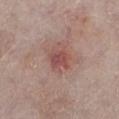Clinical impression: The lesion was photographed on a routine skin check and not biopsied; there is no pathology result. Context: The lesion is located on the left lower leg. The tile uses white-light illumination. A 15 mm close-up extracted from a 3D total-body photography capture. The recorded lesion diameter is about 3 mm. A male subject in their 80s. Automated image analysis of the tile measured border irregularity of about 2.5 on a 0–10 scale. It also reported a classifier nevus-likeness of about 5/100.